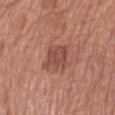Findings:
• notes: imaged on a skin check; not biopsied
• lesion diameter: about 4 mm
• site: the right thigh
• image source: ~15 mm crop, total-body skin-cancer survey
• patient: female, in their mid-70s
• automated lesion analysis: an average lesion color of about L≈48 a*≈24 b*≈28 (CIELAB) and a normalized lesion–skin contrast near 6.5; border irregularity of about 3.5 on a 0–10 scale and a color-variation rating of about 2.5/10; a classifier nevus-likeness of about 15/100 and a lesion-detection confidence of about 100/100
• lighting: white-light